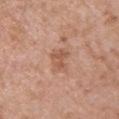Impression: This lesion was catalogued during total-body skin photography and was not selected for biopsy. Acquisition and patient details: Longest diameter approximately 3 mm. The lesion is located on the upper back. A 15 mm close-up extracted from a 3D total-body photography capture. The total-body-photography lesion software estimated a mean CIELAB color near L≈56 a*≈22 b*≈31 and a normalized border contrast of about 6. And it measured lesion-presence confidence of about 100/100. The subject is a female aged approximately 30. The tile uses white-light illumination.